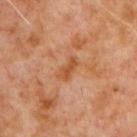Captured during whole-body skin photography for melanoma surveillance; the lesion was not biopsied. A male patient, aged approximately 60. A close-up tile cropped from a whole-body skin photograph, about 15 mm across. Measured at roughly 3 mm in maximum diameter. The lesion is located on the front of the torso. Captured under cross-polarized illumination.